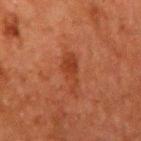This lesion was catalogued during total-body skin photography and was not selected for biopsy. Longest diameter approximately 4 mm. A close-up tile cropped from a whole-body skin photograph, about 15 mm across. A male patient, about 60 years old. The lesion is on the right upper arm. Captured under cross-polarized illumination. The total-body-photography lesion software estimated a lesion area of about 5.5 mm², an outline eccentricity of about 0.9 (0 = round, 1 = elongated), and a shape-asymmetry score of about 0.4 (0 = symmetric). It also reported a lesion color around L≈31 a*≈24 b*≈30 in CIELAB and a normalized lesion–skin contrast near 6.5. And it measured a border-irregularity index near 5/10 and a peripheral color-asymmetry measure near 1.